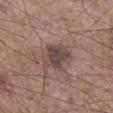The lesion was tiled from a total-body skin photograph and was not biopsied. Longest diameter approximately 4 mm. Captured under white-light illumination. The lesion-visualizer software estimated a lesion color around L≈44 a*≈16 b*≈20 in CIELAB, about 10 CIELAB-L* units darker than the surrounding skin, and a lesion-to-skin contrast of about 8.5 (normalized; higher = more distinct). The analysis additionally found border irregularity of about 3 on a 0–10 scale and a color-variation rating of about 3.5/10. The analysis additionally found a classifier nevus-likeness of about 10/100 and a lesion-detection confidence of about 100/100. A close-up tile cropped from a whole-body skin photograph, about 15 mm across. On the right lower leg. A male patient roughly 55 years of age.